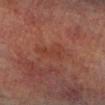Q: What is the anatomic site?
A: the left lower leg
Q: What is the lesion's diameter?
A: about 4 mm
Q: Automated lesion metrics?
A: a mean CIELAB color near L≈32 a*≈21 b*≈25 and a lesion–skin lightness drop of about 4; border irregularity of about 4 on a 0–10 scale, a within-lesion color-variation index near 1.5/10, and peripheral color asymmetry of about 0.5; a nevus-likeness score of about 0/100 and lesion-presence confidence of about 100/100
Q: What is the imaging modality?
A: total-body-photography crop, ~15 mm field of view
Q: Illumination type?
A: cross-polarized
Q: Patient demographics?
A: male, in their mid- to late 70s